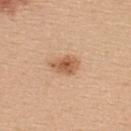lesion size: ~3.5 mm (longest diameter) | image: 15 mm crop, total-body photography | body site: the upper back | image-analysis metrics: a mean CIELAB color near L≈58 a*≈22 b*≈36; a border-irregularity index near 2.5/10, internal color variation of about 3.5 on a 0–10 scale, and peripheral color asymmetry of about 1; a classifier nevus-likeness of about 90/100 | patient: female, in their mid- to late 40s.